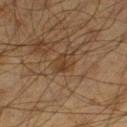This lesion was catalogued during total-body skin photography and was not selected for biopsy. Automated image analysis of the tile measured a detector confidence of about 70 out of 100 that the crop contains a lesion. Measured at roughly 3 mm in maximum diameter. Located on the leg. The patient is a male in their 60s. A roughly 15 mm field-of-view crop from a total-body skin photograph.